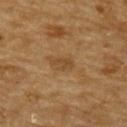– follow-up · total-body-photography surveillance lesion; no biopsy
– acquisition · total-body-photography crop, ~15 mm field of view
– subject · male, in their mid- to late 80s or older
– illumination · cross-polarized
– automated lesion analysis · a within-lesion color-variation index near 0/10 and peripheral color asymmetry of about 0
– lesion diameter · about 2.5 mm
– site · the upper back